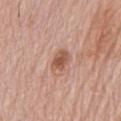| feature | finding |
|---|---|
| workup | catalogued during a skin exam; not biopsied |
| image source | 15 mm crop, total-body photography |
| site | the chest |
| subject | male, aged approximately 80 |
| lesion size | ≈3 mm |
| tile lighting | white-light |